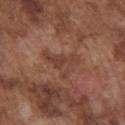| field | value |
|---|---|
| notes | imaged on a skin check; not biopsied |
| site | the front of the torso |
| lesion diameter | about 5 mm |
| patient | male, aged around 75 |
| image | 15 mm crop, total-body photography |
| tile lighting | white-light illumination |